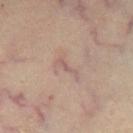– automated metrics: an area of roughly 2.5 mm² and an eccentricity of roughly 0.95; an average lesion color of about L≈56 a*≈18 b*≈22 (CIELAB), roughly 6 lightness units darker than nearby skin, and a normalized lesion–skin contrast near 5
– lesion size: ~3.5 mm (longest diameter)
– imaging modality: ~15 mm crop, total-body skin-cancer survey
– lighting: cross-polarized illumination
– patient: female, in their mid-40s
– anatomic site: the mid back